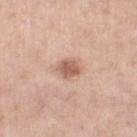biopsy status=imaged on a skin check; not biopsied | site=the right thigh | imaging modality=~15 mm crop, total-body skin-cancer survey | size=~2.5 mm (longest diameter) | subject=female, aged around 55 | automated lesion analysis=a lesion area of about 5 mm²; a mean CIELAB color near L≈59 a*≈21 b*≈28, about 13 CIELAB-L* units darker than the surrounding skin, and a normalized border contrast of about 8; a border-irregularity index near 1.5/10, a within-lesion color-variation index near 2.5/10, and peripheral color asymmetry of about 1; a nevus-likeness score of about 75/100 and lesion-presence confidence of about 100/100.